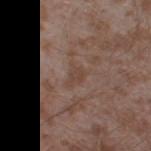Impression: This lesion was catalogued during total-body skin photography and was not selected for biopsy. Image and clinical context: Cropped from a whole-body photographic skin survey; the tile spans about 15 mm. The lesion is on the right thigh. A male patient, in their mid-40s.